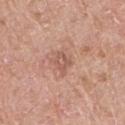Q: Is there a histopathology result?
A: total-body-photography surveillance lesion; no biopsy
Q: What kind of image is this?
A: 15 mm crop, total-body photography
Q: What are the patient's age and sex?
A: male, aged 63 to 67
Q: What lighting was used for the tile?
A: white-light illumination
Q: How large is the lesion?
A: ≈3 mm
Q: Lesion location?
A: the left upper arm